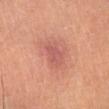Q: What kind of image is this?
A: ~15 mm tile from a whole-body skin photo
Q: Patient demographics?
A: male, about 65 years old
Q: Lesion size?
A: ~3.5 mm (longest diameter)
Q: Lesion location?
A: the left lower leg
Q: What lighting was used for the tile?
A: cross-polarized illumination